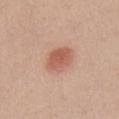diameter — about 3.5 mm | acquisition — 15 mm crop, total-body photography | anatomic site — the chest | illumination — white-light illumination | patient — female, aged 48–52 | automated metrics — an area of roughly 8.5 mm² and an outline eccentricity of about 0.55 (0 = round, 1 = elongated); a lesion color around L≈58 a*≈25 b*≈30 in CIELAB.